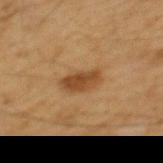Findings:
• notes — no biopsy performed (imaged during a skin exam)
• site — the mid back
• subject — male, approximately 60 years of age
• image-analysis metrics — an average lesion color of about L≈38 a*≈17 b*≈32 (CIELAB), roughly 9 lightness units darker than nearby skin, and a normalized border contrast of about 8; a nevus-likeness score of about 85/100 and a detector confidence of about 100 out of 100 that the crop contains a lesion
• imaging modality — ~15 mm tile from a whole-body skin photo
• lesion size — ≈4 mm